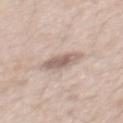This lesion was catalogued during total-body skin photography and was not selected for biopsy. The lesion is located on the mid back. The lesion's longest dimension is about 3.5 mm. A lesion tile, about 15 mm wide, cut from a 3D total-body photograph. The subject is a male aged 48–52.